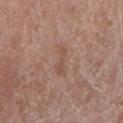follow-up = no biopsy performed (imaged during a skin exam) | automated metrics = a lesion–skin lightness drop of about 6 and a normalized border contrast of about 5 | body site = the right lower leg | image source = ~15 mm tile from a whole-body skin photo | illumination = white-light | lesion diameter = about 3.5 mm | patient = male, aged 68 to 72.